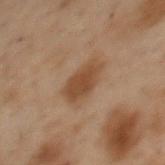A region of skin cropped from a whole-body photographic capture, roughly 15 mm wide. From the upper back. An algorithmic analysis of the crop reported a footprint of about 8.5 mm², an eccentricity of roughly 0.7, and two-axis asymmetry of about 0.2. It also reported a mean CIELAB color near L≈38 a*≈16 b*≈28, roughly 8 lightness units darker than nearby skin, and a lesion-to-skin contrast of about 8 (normalized; higher = more distinct). And it measured a border-irregularity index near 2/10, a within-lesion color-variation index near 1.5/10, and a peripheral color-asymmetry measure near 0.5. It also reported an automated nevus-likeness rating near 90 out of 100 and a lesion-detection confidence of about 100/100. Approximately 4 mm at its widest. The tile uses cross-polarized illumination. The subject is a female in their mid-50s.